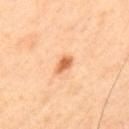Findings:
* patient · male, approximately 45 years of age
* acquisition · ~15 mm tile from a whole-body skin photo
* TBP lesion metrics · a border-irregularity index near 2.5/10, a color-variation rating of about 2.5/10, and peripheral color asymmetry of about 0.5; a nevus-likeness score of about 95/100 and a lesion-detection confidence of about 100/100
* site · the upper back
* lighting · cross-polarized
* lesion size · ~2.5 mm (longest diameter)